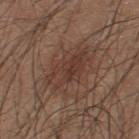Notes:
- biopsy status · no biopsy performed (imaged during a skin exam)
- acquisition · ~15 mm crop, total-body skin-cancer survey
- location · the upper back
- subject · male, roughly 25 years of age
- tile lighting · white-light illumination
- image-analysis metrics · a lesion area of about 11 mm² and a shape-asymmetry score of about 0.2 (0 = symmetric); an average lesion color of about L≈37 a*≈19 b*≈23 (CIELAB), roughly 7 lightness units darker than nearby skin, and a normalized border contrast of about 6; a lesion-detection confidence of about 100/100
- lesion size · about 5 mm